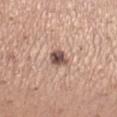* follow-up — total-body-photography surveillance lesion; no biopsy
* tile lighting — white-light
* subject — female, aged approximately 40
* image — 15 mm crop, total-body photography
* site — the right lower leg
* lesion diameter — about 2.5 mm